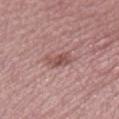Notes:
- follow-up · total-body-photography surveillance lesion; no biopsy
- lesion diameter · about 3.5 mm
- patient · female, roughly 40 years of age
- imaging modality · ~15 mm tile from a whole-body skin photo
- automated lesion analysis · a shape eccentricity near 0.85; a lesion color around L≈51 a*≈23 b*≈22 in CIELAB, a lesion–skin lightness drop of about 10, and a lesion-to-skin contrast of about 7 (normalized; higher = more distinct); a border-irregularity rating of about 3.5/10, a within-lesion color-variation index near 2.5/10, and radial color variation of about 1; a lesion-detection confidence of about 100/100
- site · the right lower leg